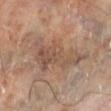follow-up: imaged on a skin check; not biopsied
site: the left lower leg
lighting: cross-polarized
subject: male, aged around 70
imaging modality: total-body-photography crop, ~15 mm field of view